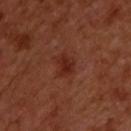The tile uses cross-polarized illumination.
Automated image analysis of the tile measured an average lesion color of about L≈27 a*≈26 b*≈27 (CIELAB), about 7 CIELAB-L* units darker than the surrounding skin, and a lesion-to-skin contrast of about 7.5 (normalized; higher = more distinct). The software also gave border irregularity of about 3.5 on a 0–10 scale and a within-lesion color-variation index near 2/10.
From the back.
The lesion's longest dimension is about 2.5 mm.
The subject is a male aged 48–52.
A 15 mm close-up tile from a total-body photography series done for melanoma screening.